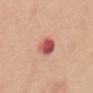No biopsy was performed on this lesion — it was imaged during a full skin examination and was not determined to be concerning.
The total-body-photography lesion software estimated an area of roughly 6.5 mm², an outline eccentricity of about 0.75 (0 = round, 1 = elongated), and a symmetry-axis asymmetry near 0.15. The analysis additionally found a mean CIELAB color near L≈55 a*≈32 b*≈26, a lesion–skin lightness drop of about 16, and a normalized border contrast of about 10.5. It also reported a border-irregularity index near 1.5/10 and peripheral color asymmetry of about 2.5. The analysis additionally found an automated nevus-likeness rating near 0 out of 100 and lesion-presence confidence of about 100/100.
A region of skin cropped from a whole-body photographic capture, roughly 15 mm wide.
The subject is a male roughly 70 years of age.
Approximately 3.5 mm at its widest.
Captured under white-light illumination.
The lesion is on the back.